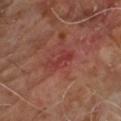lighting: cross-polarized
image:
  source: total-body photography crop
  field_of_view_mm: 15
lesion_size:
  long_diameter_mm_approx: 4.0
patient:
  sex: male
  age_approx: 65
site: chest